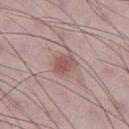patient: male, aged 48 to 52 | TBP lesion metrics: about 10 CIELAB-L* units darker than the surrounding skin | image: ~15 mm tile from a whole-body skin photo | lesion diameter: ~2.5 mm (longest diameter) | location: the left thigh | tile lighting: white-light illumination.